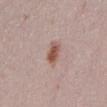workup: catalogued during a skin exam; not biopsied
patient: female, aged 28 to 32
imaging modality: total-body-photography crop, ~15 mm field of view
body site: the lower back